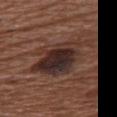This lesion was catalogued during total-body skin photography and was not selected for biopsy. A 15 mm crop from a total-body photograph taken for skin-cancer surveillance. The lesion is located on the chest. A female patient aged around 75. The recorded lesion diameter is about 7.5 mm.